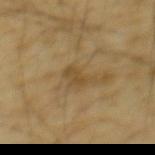Part of a total-body skin-imaging series; this lesion was reviewed on a skin check and was not flagged for biopsy.
A 15 mm close-up extracted from a 3D total-body photography capture.
Located on the mid back.
The subject is a male roughly 65 years of age.